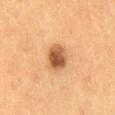Clinical impression:
The lesion was tiled from a total-body skin photograph and was not biopsied.
Background:
The patient is a female aged 38 to 42. From the back. A 15 mm close-up extracted from a 3D total-body photography capture.